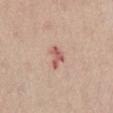Part of a total-body skin-imaging series; this lesion was reviewed on a skin check and was not flagged for biopsy. A 15 mm close-up extracted from a 3D total-body photography capture. A female subject aged 63 to 67. Captured under white-light illumination. The lesion is on the abdomen.